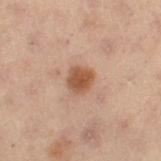Q: Is there a histopathology result?
A: total-body-photography surveillance lesion; no biopsy
Q: What is the anatomic site?
A: the left thigh
Q: What kind of image is this?
A: ~15 mm crop, total-body skin-cancer survey
Q: Patient demographics?
A: female, in their mid- to late 50s